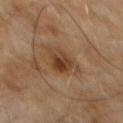image source: ~15 mm crop, total-body skin-cancer survey | patient: male, aged 58 to 62 | anatomic site: the abdomen.